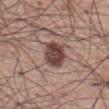  biopsy_status: not biopsied; imaged during a skin examination
  image:
    source: total-body photography crop
    field_of_view_mm: 15
  lighting: white-light
  site: abdomen
  automated_metrics:
    area_mm2_approx: 9.0
    eccentricity: 0.45
    nevus_likeness_0_100: 90
    lesion_detection_confidence_0_100: 100
  lesion_size:
    long_diameter_mm_approx: 3.5
  patient:
    sex: male
    age_approx: 60